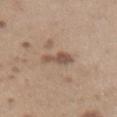Q: Was this lesion biopsied?
A: catalogued during a skin exam; not biopsied
Q: What is the anatomic site?
A: the abdomen
Q: How large is the lesion?
A: ≈3.5 mm
Q: What are the patient's age and sex?
A: female, in their mid- to late 50s
Q: What kind of image is this?
A: ~15 mm crop, total-body skin-cancer survey